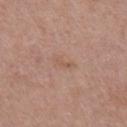Findings:
* body site: the front of the torso
* patient: male, about 55 years old
* image source: 15 mm crop, total-body photography